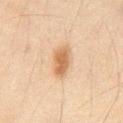Impression: Part of a total-body skin-imaging series; this lesion was reviewed on a skin check and was not flagged for biopsy. Context: The tile uses cross-polarized illumination. A male patient, aged around 65. A 15 mm close-up tile from a total-body photography series done for melanoma screening. The lesion is located on the abdomen.